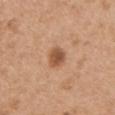Imaged during a routine full-body skin examination; the lesion was not biopsied and no histopathology is available. From the chest. A region of skin cropped from a whole-body photographic capture, roughly 15 mm wide. The subject is a male aged 48 to 52. Approximately 2.5 mm at its widest. Imaged with white-light lighting.